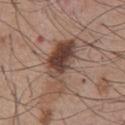Imaged during a routine full-body skin examination; the lesion was not biopsied and no histopathology is available.
The total-body-photography lesion software estimated a lesion area of about 18 mm², an outline eccentricity of about 0.85 (0 = round, 1 = elongated), and two-axis asymmetry of about 0.5. And it measured about 12 CIELAB-L* units darker than the surrounding skin and a normalized border contrast of about 9.5. The analysis additionally found a border-irregularity index near 6.5/10, a color-variation rating of about 9/10, and radial color variation of about 3.
Cropped from a whole-body photographic skin survey; the tile spans about 15 mm.
The lesion is located on the chest.
The lesion's longest dimension is about 7 mm.
The patient is a male in their mid- to late 50s.